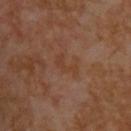Part of a total-body skin-imaging series; this lesion was reviewed on a skin check and was not flagged for biopsy.